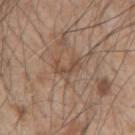notes=catalogued during a skin exam; not biopsied | lesion diameter=≈3 mm | image=15 mm crop, total-body photography | automated lesion analysis=an area of roughly 3.5 mm² and two-axis asymmetry of about 0.6; a mean CIELAB color near L≈48 a*≈17 b*≈28, a lesion–skin lightness drop of about 7, and a normalized border contrast of about 5.5; an automated nevus-likeness rating near 0 out of 100 and a lesion-detection confidence of about 80/100 | subject=male, aged approximately 50 | lighting=white-light illumination | site=the right upper arm.